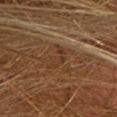Q: Who is the patient?
A: female, aged 53 to 57
Q: Illumination type?
A: cross-polarized
Q: Lesion location?
A: the chest
Q: What is the lesion's diameter?
A: about 3 mm
Q: What did automated image analysis measure?
A: about 6 CIELAB-L* units darker than the surrounding skin and a lesion-to-skin contrast of about 5.5 (normalized; higher = more distinct); a border-irregularity rating of about 6.5/10 and a color-variation rating of about 0/10
Q: How was this image acquired?
A: total-body-photography crop, ~15 mm field of view